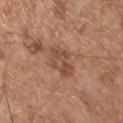workup: no biopsy performed (imaged during a skin exam) | patient: male, in their mid- to late 50s | tile lighting: white-light | anatomic site: the chest | imaging modality: ~15 mm tile from a whole-body skin photo | image-analysis metrics: a lesion area of about 7.5 mm², an outline eccentricity of about 0.85 (0 = round, 1 = elongated), and two-axis asymmetry of about 0.3; a color-variation rating of about 3/10 and peripheral color asymmetry of about 1; an automated nevus-likeness rating near 0 out of 100 and a detector confidence of about 100 out of 100 that the crop contains a lesion.